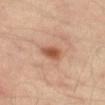Case summary:
• workup — catalogued during a skin exam; not biopsied
• location — the left thigh
• subject — male, roughly 55 years of age
• image source — 15 mm crop, total-body photography